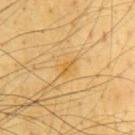Assessment:
Part of a total-body skin-imaging series; this lesion was reviewed on a skin check and was not flagged for biopsy.
Background:
Automated tile analysis of the lesion measured an area of roughly 3 mm² and an outline eccentricity of about 0.85 (0 = round, 1 = elongated). The software also gave a border-irregularity rating of about 2/10 and peripheral color asymmetry of about 1. A male patient, approximately 65 years of age. Cropped from a total-body skin-imaging series; the visible field is about 15 mm. The lesion is located on the chest. The tile uses cross-polarized illumination.